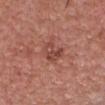Impression:
This lesion was catalogued during total-body skin photography and was not selected for biopsy.
Clinical summary:
Located on the chest. The subject is a male aged 58–62. A 15 mm close-up tile from a total-body photography series done for melanoma screening. Approximately 3 mm at its widest. The lesion-visualizer software estimated an average lesion color of about L≈46 a*≈26 b*≈28 (CIELAB), about 8 CIELAB-L* units darker than the surrounding skin, and a lesion-to-skin contrast of about 6.5 (normalized; higher = more distinct). It also reported a color-variation rating of about 4/10 and a peripheral color-asymmetry measure near 1.5.